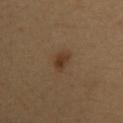The lesion was photographed on a routine skin check and not biopsied; there is no pathology result. The patient is a female aged 33 to 37. A region of skin cropped from a whole-body photographic capture, roughly 15 mm wide. From the arm.